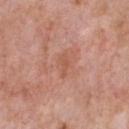imaging modality=15 mm crop, total-body photography
patient=male, aged 58 to 62
body site=the front of the torso
illumination=white-light illumination